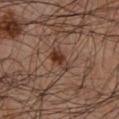No biopsy was performed on this lesion — it was imaged during a full skin examination and was not determined to be concerning.
The lesion-visualizer software estimated a lesion area of about 5 mm², a shape eccentricity near 0.8, and a symmetry-axis asymmetry near 0.35. It also reported a nevus-likeness score of about 90/100.
The subject is a male in their mid- to late 40s.
Cropped from a total-body skin-imaging series; the visible field is about 15 mm.
Located on the arm.